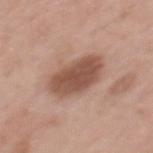Clinical impression: Imaged during a routine full-body skin examination; the lesion was not biopsied and no histopathology is available. Context: The lesion is located on the upper back. A close-up tile cropped from a whole-body skin photograph, about 15 mm across. The subject is a female aged 48 to 52.